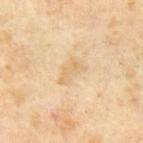A 15 mm close-up tile from a total-body photography series done for melanoma screening. The tile uses cross-polarized illumination. The lesion's longest dimension is about 3.5 mm. The total-body-photography lesion software estimated a lesion area of about 5.5 mm² and a symmetry-axis asymmetry near 0.35. The software also gave a border-irregularity rating of about 4/10, a color-variation rating of about 1.5/10, and a peripheral color-asymmetry measure near 0.5. It also reported a classifier nevus-likeness of about 0/100. A male subject, about 65 years old.